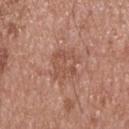Captured during whole-body skin photography for melanoma surveillance; the lesion was not biopsied. A male subject, aged around 55. Cropped from a total-body skin-imaging series; the visible field is about 15 mm. Measured at roughly 4 mm in maximum diameter. The lesion is on the upper back. Automated tile analysis of the lesion measured a shape eccentricity near 0.6.